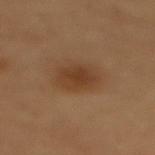– workup — total-body-photography surveillance lesion; no biopsy
– automated lesion analysis — border irregularity of about 1.5 on a 0–10 scale, internal color variation of about 2 on a 0–10 scale, and radial color variation of about 0.5
– acquisition — ~15 mm tile from a whole-body skin photo
– illumination — cross-polarized
– patient — female, in their 50s
– site — the upper back